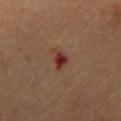Recorded during total-body skin imaging; not selected for excision or biopsy.
The lesion is on the mid back.
A male patient aged around 55.
The total-body-photography lesion software estimated an area of roughly 3 mm², an eccentricity of roughly 0.8, and a symmetry-axis asymmetry near 0.3.
A region of skin cropped from a whole-body photographic capture, roughly 15 mm wide.
Approximately 2.5 mm at its widest.